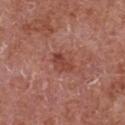The lesion was tiled from a total-body skin photograph and was not biopsied.
The subject is a male about 65 years old.
Approximately 3 mm at its widest.
From the chest.
Cropped from a whole-body photographic skin survey; the tile spans about 15 mm.